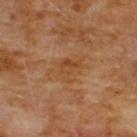| key | value |
|---|---|
| biopsy status | no biopsy performed (imaged during a skin exam) |
| anatomic site | the chest |
| patient | male, aged approximately 60 |
| acquisition | ~15 mm tile from a whole-body skin photo |